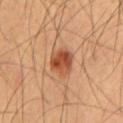Impression: The lesion was tiled from a total-body skin photograph and was not biopsied. Image and clinical context: About 3.5 mm across. Imaged with cross-polarized lighting. On the chest. A close-up tile cropped from a whole-body skin photograph, about 15 mm across. A male patient in their mid-50s. The lesion-visualizer software estimated a lesion color around L≈48 a*≈26 b*≈35 in CIELAB and roughly 13 lightness units darker than nearby skin. And it measured border irregularity of about 1.5 on a 0–10 scale and peripheral color asymmetry of about 2.